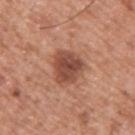  image:
    source: total-body photography crop
    field_of_view_mm: 15
  patient:
    sex: male
    age_approx: 55
  lesion_size:
    long_diameter_mm_approx: 4.0
  site: upper back